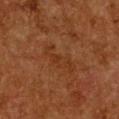Case summary:
– biopsy status — catalogued during a skin exam; not biopsied
– subject — female, in their 50s
– location — the chest
– image — 15 mm crop, total-body photography